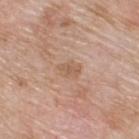Clinical impression:
This lesion was catalogued during total-body skin photography and was not selected for biopsy.
Acquisition and patient details:
The lesion is located on the upper back. A 15 mm close-up extracted from a 3D total-body photography capture. Measured at roughly 2.5 mm in maximum diameter. Automated tile analysis of the lesion measured a lesion area of about 3.5 mm², an eccentricity of roughly 0.75, and a symmetry-axis asymmetry near 0.3. And it measured a lesion-detection confidence of about 100/100. The patient is a male aged 58 to 62.